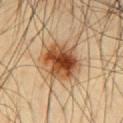location = the chest
image source = ~15 mm tile from a whole-body skin photo
tile lighting = cross-polarized illumination
lesion diameter = ~4.5 mm (longest diameter)
patient = male, about 45 years old